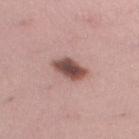Clinical impression:
Part of a total-body skin-imaging series; this lesion was reviewed on a skin check and was not flagged for biopsy.
Acquisition and patient details:
The tile uses white-light illumination. A roughly 15 mm field-of-view crop from a total-body skin photograph. A female patient, approximately 25 years of age. On the left thigh.